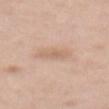Captured during whole-body skin photography for melanoma surveillance; the lesion was not biopsied.
The lesion is on the abdomen.
Cropped from a whole-body photographic skin survey; the tile spans about 15 mm.
Longest diameter approximately 3 mm.
A female subject, aged approximately 40.